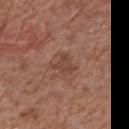biopsy_status: not biopsied; imaged during a skin examination
site: chest
patient:
  sex: male
  age_approx: 75
automated_metrics:
  area_mm2_approx: 4.0
  eccentricity: 0.45
  cielab_L: 43
  cielab_a: 22
  cielab_b: 26
  vs_skin_darker_L: 7.0
  vs_skin_contrast_norm: 5.5
  color_variation_0_10: 1.5
image:
  source: total-body photography crop
  field_of_view_mm: 15
lighting: white-light